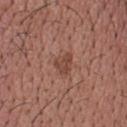Notes:
* follow-up: total-body-photography surveillance lesion; no biopsy
* illumination: white-light
* lesion size: ≈2.5 mm
* anatomic site: the head or neck
* acquisition: ~15 mm tile from a whole-body skin photo
* subject: male, aged around 55